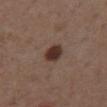This lesion was catalogued during total-body skin photography and was not selected for biopsy.
Captured under white-light illumination.
The subject is a male about 55 years old.
From the abdomen.
The recorded lesion diameter is about 2.5 mm.
A 15 mm close-up tile from a total-body photography series done for melanoma screening.